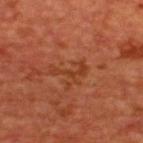follow-up=catalogued during a skin exam; not biopsied
location=the back
imaging modality=~15 mm tile from a whole-body skin photo
subject=male, in their mid-60s